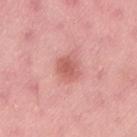notes: catalogued during a skin exam; not biopsied | size: ~3 mm (longest diameter) | illumination: white-light illumination | subject: female, aged 48–52 | site: the lower back | imaging modality: total-body-photography crop, ~15 mm field of view.